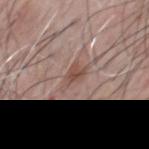Assessment:
The lesion was photographed on a routine skin check and not biopsied; there is no pathology result.
Image and clinical context:
From the front of the torso. A 15 mm crop from a total-body photograph taken for skin-cancer surveillance. A male subject, aged around 65.